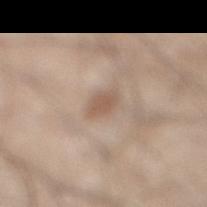* illumination: white-light
* location: the right lower leg
* image: total-body-photography crop, ~15 mm field of view
* patient: male, about 45 years old